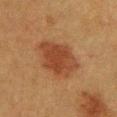  biopsy_status: not biopsied; imaged during a skin examination
  site: chest
  patient:
    sex: female
    age_approx: 40
  lighting: cross-polarized
  automated_metrics:
    cielab_L: 35
    cielab_a: 21
    cielab_b: 30
    vs_skin_darker_L: 9.0
    vs_skin_contrast_norm: 8.0
  lesion_size:
    long_diameter_mm_approx: 6.0
  image:
    source: total-body photography crop
    field_of_view_mm: 15